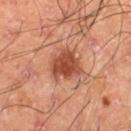lesion size=≈3.5 mm
patient=male, aged around 60
site=the left thigh
image=~15 mm tile from a whole-body skin photo